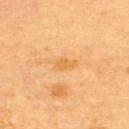Impression: Imaged during a routine full-body skin examination; the lesion was not biopsied and no histopathology is available. Clinical summary: The lesion-visualizer software estimated a border-irregularity index near 2.5/10 and a within-lesion color-variation index near 0.5/10. It also reported a classifier nevus-likeness of about 0/100 and a detector confidence of about 100 out of 100 that the crop contains a lesion. The patient is a female aged 53–57. Measured at roughly 2.5 mm in maximum diameter. Captured under cross-polarized illumination. From the upper back. Cropped from a whole-body photographic skin survey; the tile spans about 15 mm.